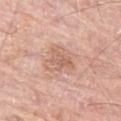notes: no biopsy performed (imaged during a skin exam)
imaging modality: 15 mm crop, total-body photography
body site: the left thigh
patient: male, approximately 80 years of age